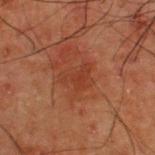follow-up: catalogued during a skin exam; not biopsied
image: total-body-photography crop, ~15 mm field of view
site: the upper back
automated metrics: an area of roughly 7 mm², a shape eccentricity near 0.75, and a shape-asymmetry score of about 0.35 (0 = symmetric); a mean CIELAB color near L≈29 a*≈22 b*≈26 and about 5 CIELAB-L* units darker than the surrounding skin; a border-irregularity index near 3.5/10, a color-variation rating of about 1.5/10, and peripheral color asymmetry of about 0.5; an automated nevus-likeness rating near 0 out of 100 and a lesion-detection confidence of about 100/100
diameter: ≈3.5 mm
illumination: cross-polarized illumination
patient: male, aged around 50